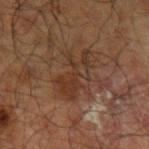follow-up: imaged on a skin check; not biopsied
subject: male, aged approximately 70
anatomic site: the left upper arm
image source: ~15 mm crop, total-body skin-cancer survey